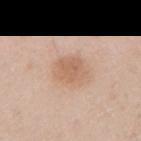Recorded during total-body skin imaging; not selected for excision or biopsy. On the right upper arm. A lesion tile, about 15 mm wide, cut from a 3D total-body photograph. A female subject roughly 40 years of age. This is a white-light tile. Automated image analysis of the tile measured a within-lesion color-variation index near 2.5/10 and a peripheral color-asymmetry measure near 1. It also reported a nevus-likeness score of about 80/100 and a detector confidence of about 100 out of 100 that the crop contains a lesion.